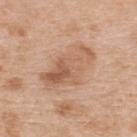Findings:
– follow-up — imaged on a skin check; not biopsied
– tile lighting — white-light
– location — the upper back
– patient — male, about 70 years old
– acquisition — total-body-photography crop, ~15 mm field of view
– TBP lesion metrics — an outline eccentricity of about 0.85 (0 = round, 1 = elongated) and two-axis asymmetry of about 0.2; about 9 CIELAB-L* units darker than the surrounding skin and a lesion-to-skin contrast of about 6 (normalized; higher = more distinct)
– size — ~7 mm (longest diameter)